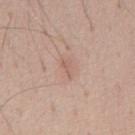Impression: Recorded during total-body skin imaging; not selected for excision or biopsy. Image and clinical context: The recorded lesion diameter is about 2.5 mm. The lesion is on the abdomen. The tile uses white-light illumination. A roughly 15 mm field-of-view crop from a total-body skin photograph. The subject is a male aged around 45.